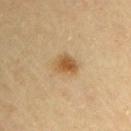The lesion was tiled from a total-body skin photograph and was not biopsied. The lesion is on the left upper arm. A 15 mm close-up tile from a total-body photography series done for melanoma screening. Longest diameter approximately 3 mm. An algorithmic analysis of the crop reported a footprint of about 6 mm² and an outline eccentricity of about 0.55 (0 = round, 1 = elongated). It also reported a mean CIELAB color near L≈49 a*≈15 b*≈35, a lesion–skin lightness drop of about 10, and a normalized lesion–skin contrast near 8. The tile uses cross-polarized illumination. A male patient aged approximately 60.